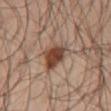illumination: cross-polarized; subject: male, approximately 50 years of age; diameter: ~5.5 mm (longest diameter); acquisition: ~15 mm crop, total-body skin-cancer survey; site: the front of the torso.